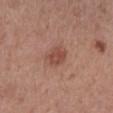{"biopsy_status": "not biopsied; imaged during a skin examination", "lighting": "white-light", "automated_metrics": {"border_irregularity_0_10": 2.0, "color_variation_0_10": 3.0, "peripheral_color_asymmetry": 1.5, "nevus_likeness_0_100": 55, "lesion_detection_confidence_0_100": 100}, "image": {"source": "total-body photography crop", "field_of_view_mm": 15}, "lesion_size": {"long_diameter_mm_approx": 3.0}, "site": "leg", "patient": {"sex": "female", "age_approx": 65}}